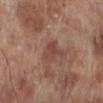Clinical impression: No biopsy was performed on this lesion — it was imaged during a full skin examination and was not determined to be concerning. Acquisition and patient details: A male subject, aged 68–72. A 15 mm crop from a total-body photograph taken for skin-cancer surveillance. Located on the leg. About 3 mm across.